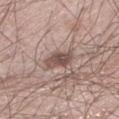{
  "biopsy_status": "not biopsied; imaged during a skin examination",
  "site": "left lower leg",
  "image": {
    "source": "total-body photography crop",
    "field_of_view_mm": 15
  },
  "lesion_size": {
    "long_diameter_mm_approx": 3.5
  },
  "automated_metrics": {
    "eccentricity": 0.85,
    "vs_skin_darker_L": 12.0,
    "vs_skin_contrast_norm": 8.5,
    "border_irregularity_0_10": 3.0
  },
  "patient": {
    "sex": "male",
    "age_approx": 40
  },
  "lighting": "white-light"
}